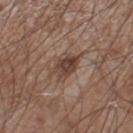Imaged during a routine full-body skin examination; the lesion was not biopsied and no histopathology is available.
The patient is a male aged 53 to 57.
The total-body-photography lesion software estimated a lesion area of about 6 mm², an outline eccentricity of about 0.75 (0 = round, 1 = elongated), and a symmetry-axis asymmetry near 0.2. It also reported a mean CIELAB color near L≈41 a*≈17 b*≈25, about 11 CIELAB-L* units darker than the surrounding skin, and a normalized lesion–skin contrast near 9. The analysis additionally found a classifier nevus-likeness of about 60/100 and lesion-presence confidence of about 100/100.
Longest diameter approximately 3.5 mm.
From the right lower leg.
A 15 mm close-up extracted from a 3D total-body photography capture.
This is a white-light tile.